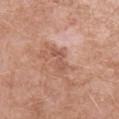<case>
  <biopsy_status>not biopsied; imaged during a skin examination</biopsy_status>
  <site>left forearm</site>
  <lesion_size>
    <long_diameter_mm_approx>3.0</long_diameter_mm_approx>
  </lesion_size>
  <automated_metrics>
    <area_mm2_approx>3.0</area_mm2_approx>
    <eccentricity>0.95</eccentricity>
    <shape_asymmetry>0.5</shape_asymmetry>
    <cielab_L>54</cielab_L>
    <cielab_a>23</cielab_a>
    <cielab_b>29</cielab_b>
    <vs_skin_contrast_norm>6.0</vs_skin_contrast_norm>
  </automated_metrics>
  <patient>
    <sex>female</sex>
    <age_approx>55</age_approx>
  </patient>
  <lighting>white-light</lighting>
  <image>
    <source>total-body photography crop</source>
    <field_of_view_mm>15</field_of_view_mm>
  </image>
</case>